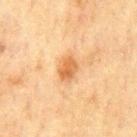Impression: Recorded during total-body skin imaging; not selected for excision or biopsy. Clinical summary: A male patient aged 68 to 72. Automated tile analysis of the lesion measured an area of roughly 5.5 mm², an outline eccentricity of about 0.7 (0 = round, 1 = elongated), and a shape-asymmetry score of about 0.2 (0 = symmetric). It also reported an automated nevus-likeness rating near 85 out of 100. A 15 mm close-up tile from a total-body photography series done for melanoma screening. The lesion is on the mid back. The tile uses cross-polarized illumination.